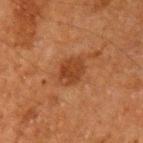Impression:
Recorded during total-body skin imaging; not selected for excision or biopsy.
Background:
A male subject, about 60 years old. On the arm. Automated image analysis of the tile measured an eccentricity of roughly 0.65. The analysis additionally found internal color variation of about 2.5 on a 0–10 scale and radial color variation of about 0.5. The software also gave a classifier nevus-likeness of about 15/100 and a lesion-detection confidence of about 100/100. Cropped from a whole-body photographic skin survey; the tile spans about 15 mm.